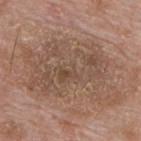Q: Was this lesion biopsied?
A: no biopsy performed (imaged during a skin exam)
Q: How large is the lesion?
A: about 11.5 mm
Q: What are the patient's age and sex?
A: male, roughly 60 years of age
Q: Where on the body is the lesion?
A: the upper back
Q: What is the imaging modality?
A: total-body-photography crop, ~15 mm field of view
Q: How was the tile lit?
A: white-light illumination